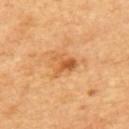workup: total-body-photography surveillance lesion; no biopsy
acquisition: total-body-photography crop, ~15 mm field of view
TBP lesion metrics: an outline eccentricity of about 0.6 (0 = round, 1 = elongated) and two-axis asymmetry of about 0.5; an automated nevus-likeness rating near 35 out of 100 and a detector confidence of about 100 out of 100 that the crop contains a lesion
subject: male, approximately 65 years of age
lesion diameter: about 3 mm
tile lighting: cross-polarized illumination
site: the mid back